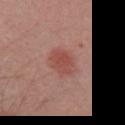  biopsy_status: not biopsied; imaged during a skin examination
  site: chest
  image:
    source: total-body photography crop
    field_of_view_mm: 15
  patient:
    sex: male
    age_approx: 60